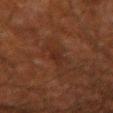The lesion was tiled from a total-body skin photograph and was not biopsied. The lesion is on the left forearm. The lesion's longest dimension is about 2.5 mm. This is a cross-polarized tile. Cropped from a total-body skin-imaging series; the visible field is about 15 mm. A male patient, aged around 60.